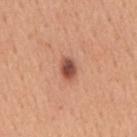This lesion was catalogued during total-body skin photography and was not selected for biopsy.
On the back.
Cropped from a total-body skin-imaging series; the visible field is about 15 mm.
The lesion's longest dimension is about 2.5 mm.
The tile uses white-light illumination.
The lesion-visualizer software estimated a lesion color around L≈51 a*≈26 b*≈29 in CIELAB, a lesion–skin lightness drop of about 16, and a normalized lesion–skin contrast near 10.5. The software also gave border irregularity of about 1.5 on a 0–10 scale, a color-variation rating of about 5/10, and radial color variation of about 1.5.
A male subject in their mid-50s.